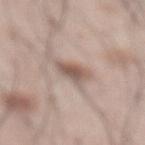Assessment: The lesion was photographed on a routine skin check and not biopsied; there is no pathology result. Image and clinical context: On the abdomen. A close-up tile cropped from a whole-body skin photograph, about 15 mm across. Approximately 3 mm at its widest. A male patient in their mid-50s. An algorithmic analysis of the crop reported a mean CIELAB color near L≈55 a*≈16 b*≈23, about 12 CIELAB-L* units darker than the surrounding skin, and a normalized lesion–skin contrast near 8. The analysis additionally found a border-irregularity index near 3/10, a within-lesion color-variation index near 3.5/10, and radial color variation of about 1. It also reported a nevus-likeness score of about 75/100 and lesion-presence confidence of about 100/100.